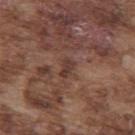The lesion was photographed on a routine skin check and not biopsied; there is no pathology result. This is a white-light tile. The lesion's longest dimension is about 2.5 mm. Cropped from a whole-body photographic skin survey; the tile spans about 15 mm. On the mid back. Automated tile analysis of the lesion measured a shape eccentricity near 0.8 and a shape-asymmetry score of about 0.3 (0 = symmetric). The analysis additionally found radial color variation of about 0.5. The software also gave a nevus-likeness score of about 0/100 and a lesion-detection confidence of about 55/100. A male subject aged 73 to 77.